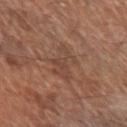{"biopsy_status": "not biopsied; imaged during a skin examination", "site": "left forearm", "patient": {"sex": "male", "age_approx": 70}, "lighting": "white-light", "lesion_size": {"long_diameter_mm_approx": 5.0}, "image": {"source": "total-body photography crop", "field_of_view_mm": 15}}